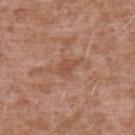Acquisition and patient details: Imaged with white-light lighting. About 3 mm across. The subject is a male aged around 45. Located on the upper back. Cropped from a total-body skin-imaging series; the visible field is about 15 mm.